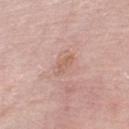Q: Was a biopsy performed?
A: imaged on a skin check; not biopsied
Q: Illumination type?
A: white-light
Q: Patient demographics?
A: male, roughly 70 years of age
Q: How was this image acquired?
A: total-body-photography crop, ~15 mm field of view
Q: How large is the lesion?
A: ≈3 mm
Q: Lesion location?
A: the left lower leg